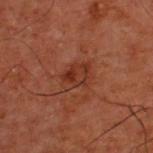{
  "biopsy_status": "not biopsied; imaged during a skin examination"
}